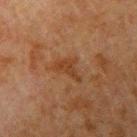workup: catalogued during a skin exam; not biopsied | site: the left upper arm | lighting: cross-polarized | imaging modality: ~15 mm tile from a whole-body skin photo | image-analysis metrics: an area of roughly 4.5 mm², a shape eccentricity near 0.8, and two-axis asymmetry of about 0.65; a border-irregularity index near 7/10, a within-lesion color-variation index near 0.5/10, and peripheral color asymmetry of about 0; a classifier nevus-likeness of about 0/100 and a lesion-detection confidence of about 100/100 | subject: male, in their 80s | size: ~3.5 mm (longest diameter).